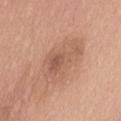Assessment:
Recorded during total-body skin imaging; not selected for excision or biopsy.
Acquisition and patient details:
The lesion is located on the abdomen. A female subject approximately 60 years of age. A close-up tile cropped from a whole-body skin photograph, about 15 mm across.